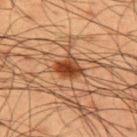Captured during whole-body skin photography for melanoma surveillance; the lesion was not biopsied. A close-up tile cropped from a whole-body skin photograph, about 15 mm across. The subject is a male aged around 60. On the upper back. The tile uses cross-polarized illumination.